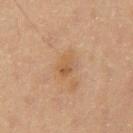Notes:
– patient: male, aged approximately 60
– imaging modality: total-body-photography crop, ~15 mm field of view